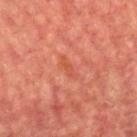Approximately 2.5 mm at its widest. A male subject, aged around 60. Located on the chest. A lesion tile, about 15 mm wide, cut from a 3D total-body photograph.